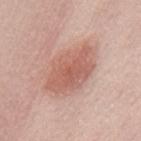workup: no biopsy performed (imaged during a skin exam) | location: the mid back | lesion size: about 7 mm | subject: female, aged around 50 | imaging modality: 15 mm crop, total-body photography | lighting: white-light illumination | image-analysis metrics: a footprint of about 20 mm², an eccentricity of roughly 0.8, and two-axis asymmetry of about 0.2; a border-irregularity rating of about 2.5/10, internal color variation of about 3.5 on a 0–10 scale, and radial color variation of about 1.